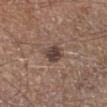notes=catalogued during a skin exam; not biopsied
lighting=white-light illumination
subject=male, aged approximately 65
imaging modality=15 mm crop, total-body photography
site=the right lower leg
lesion size=about 3 mm